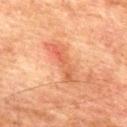{"biopsy_status": "not biopsied; imaged during a skin examination", "patient": {"sex": "male", "age_approx": 75}, "image": {"source": "total-body photography crop", "field_of_view_mm": 15}, "automated_metrics": {"border_irregularity_0_10": 5.5, "color_variation_0_10": 6.5, "peripheral_color_asymmetry": 2.5}, "lighting": "cross-polarized", "site": "mid back"}